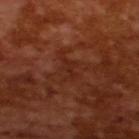The lesion was tiled from a total-body skin photograph and was not biopsied. A close-up tile cropped from a whole-body skin photograph, about 15 mm across. The subject is a male aged approximately 65. Measured at roughly 2.5 mm in maximum diameter.